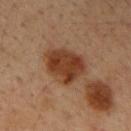{"automated_metrics": {"border_irregularity_0_10": 2.0, "color_variation_0_10": 4.0, "peripheral_color_asymmetry": 1.5, "nevus_likeness_0_100": 75, "lesion_detection_confidence_0_100": 100}, "lesion_size": {"long_diameter_mm_approx": 5.5}, "lighting": "cross-polarized", "image": {"source": "total-body photography crop", "field_of_view_mm": 15}, "patient": {"sex": "male", "age_approx": 35}, "site": "upper back"}